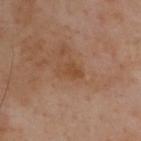– anatomic site: the upper back
– imaging modality: total-body-photography crop, ~15 mm field of view
– subject: male, aged around 55